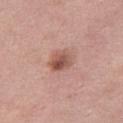This lesion was catalogued during total-body skin photography and was not selected for biopsy. The subject is a female about 50 years old. A close-up tile cropped from a whole-body skin photograph, about 15 mm across. The recorded lesion diameter is about 3 mm. On the right thigh. Automated image analysis of the tile measured a footprint of about 6.5 mm², an eccentricity of roughly 0.55, and a symmetry-axis asymmetry near 0.15. The software also gave a lesion-detection confidence of about 100/100.